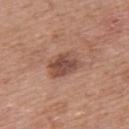biopsy status: total-body-photography surveillance lesion; no biopsy | illumination: white-light | site: the upper back | imaging modality: total-body-photography crop, ~15 mm field of view | patient: female, about 60 years old | lesion diameter: ≈4 mm.